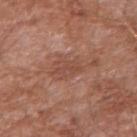<tbp_lesion>
  <biopsy_status>not biopsied; imaged during a skin examination</biopsy_status>
  <image>
    <source>total-body photography crop</source>
    <field_of_view_mm>15</field_of_view_mm>
  </image>
  <patient>
    <sex>male</sex>
    <age_approx>60</age_approx>
  </patient>
  <lighting>white-light</lighting>
  <lesion_size>
    <long_diameter_mm_approx>3.0</long_diameter_mm_approx>
  </lesion_size>
  <site>arm</site>
</tbp_lesion>